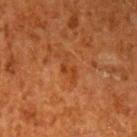Assessment:
Imaged during a routine full-body skin examination; the lesion was not biopsied and no histopathology is available.
Acquisition and patient details:
From the left upper arm. This is a cross-polarized tile. A close-up tile cropped from a whole-body skin photograph, about 15 mm across. The lesion's longest dimension is about 3 mm. The subject is a male approximately 60 years of age. An algorithmic analysis of the crop reported a detector confidence of about 100 out of 100 that the crop contains a lesion.